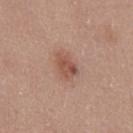Impression:
No biopsy was performed on this lesion — it was imaged during a full skin examination and was not determined to be concerning.
Acquisition and patient details:
A male subject aged approximately 25. From the chest. Cropped from a whole-body photographic skin survey; the tile spans about 15 mm.